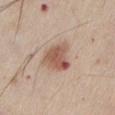biopsy status: catalogued during a skin exam; not biopsied
subject: male, aged around 45
body site: the chest
acquisition: ~15 mm tile from a whole-body skin photo